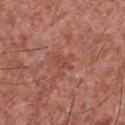{"biopsy_status": "not biopsied; imaged during a skin examination", "patient": {"sex": "male", "age_approx": 45}, "site": "front of the torso", "lighting": "white-light", "automated_metrics": {"cielab_L": 47, "cielab_a": 27, "cielab_b": 29, "vs_skin_darker_L": 6.0, "vs_skin_contrast_norm": 5.0, "nevus_likeness_0_100": 0}, "image": {"source": "total-body photography crop", "field_of_view_mm": 15}, "lesion_size": {"long_diameter_mm_approx": 2.5}}